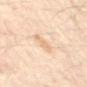follow-up = catalogued during a skin exam; not biopsied | image = 15 mm crop, total-body photography | tile lighting = cross-polarized | site = the mid back | automated metrics = a mean CIELAB color near L≈75 a*≈17 b*≈35 and a lesion–skin lightness drop of about 7; border irregularity of about 4.5 on a 0–10 scale, a color-variation rating of about 1.5/10, and peripheral color asymmetry of about 0.5; a nevus-likeness score of about 0/100 and lesion-presence confidence of about 100/100 | size = ~3.5 mm (longest diameter) | patient = aged approximately 55.